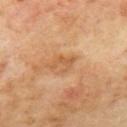{
  "biopsy_status": "not biopsied; imaged during a skin examination",
  "patient": {
    "sex": "male",
    "age_approx": 70
  },
  "image": {
    "source": "total-body photography crop",
    "field_of_view_mm": 15
  },
  "site": "mid back",
  "lesion_size": {
    "long_diameter_mm_approx": 3.0
  },
  "lighting": "cross-polarized",
  "automated_metrics": {
    "border_irregularity_0_10": 6.5,
    "peripheral_color_asymmetry": 0.0,
    "nevus_likeness_0_100": 0,
    "lesion_detection_confidence_0_100": 100
  }
}